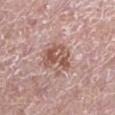workup: imaged on a skin check; not biopsied
illumination: white-light
automated lesion analysis: a lesion area of about 9 mm², a shape eccentricity near 0.65, and two-axis asymmetry of about 0.4; a classifier nevus-likeness of about 35/100 and a detector confidence of about 100 out of 100 that the crop contains a lesion
patient: male, roughly 50 years of age
location: the leg
imaging modality: total-body-photography crop, ~15 mm field of view
size: ≈4 mm